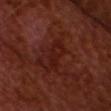biopsy status: no biopsy performed (imaged during a skin exam)
size: about 4.5 mm
image source: ~15 mm crop, total-body skin-cancer survey
lighting: cross-polarized illumination
TBP lesion metrics: a lesion area of about 6.5 mm², an outline eccentricity of about 0.9 (0 = round, 1 = elongated), and two-axis asymmetry of about 0.35; roughly 5 lightness units darker than nearby skin and a normalized border contrast of about 6; a within-lesion color-variation index near 2/10 and radial color variation of about 0.5; a lesion-detection confidence of about 90/100
subject: male, approximately 65 years of age